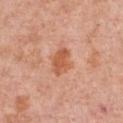Notes:
- follow-up · imaged on a skin check; not biopsied
- subject · female, aged 58 to 62
- image · 15 mm crop, total-body photography
- lesion diameter · about 3.5 mm
- illumination · white-light illumination
- site · the front of the torso